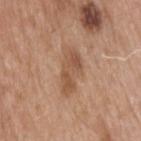Impression:
The lesion was tiled from a total-body skin photograph and was not biopsied.
Clinical summary:
The tile uses white-light illumination. Cropped from a total-body skin-imaging series; the visible field is about 15 mm. Located on the upper back. A male patient, in their mid-60s.